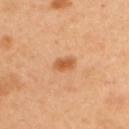Cropped from a whole-body photographic skin survey; the tile spans about 15 mm. Located on the upper back. A female patient, aged 38–42. The total-body-photography lesion software estimated a lesion area of about 4 mm² and an eccentricity of roughly 0.75. And it measured a mean CIELAB color near L≈59 a*≈26 b*≈42, roughly 11 lightness units darker than nearby skin, and a lesion-to-skin contrast of about 8 (normalized; higher = more distinct). And it measured a border-irregularity rating of about 2/10 and a color-variation rating of about 2/10. And it measured an automated nevus-likeness rating near 95 out of 100 and a detector confidence of about 100 out of 100 that the crop contains a lesion. Longest diameter approximately 2.5 mm.